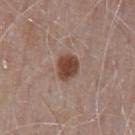Assessment:
Part of a total-body skin-imaging series; this lesion was reviewed on a skin check and was not flagged for biopsy.
Image and clinical context:
A region of skin cropped from a whole-body photographic capture, roughly 15 mm wide. The lesion is located on the front of the torso. The tile uses white-light illumination. Measured at roughly 3 mm in maximum diameter. The patient is a male aged approximately 75.